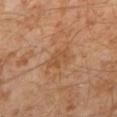workup: total-body-photography surveillance lesion; no biopsy
image-analysis metrics: a detector confidence of about 100 out of 100 that the crop contains a lesion
location: the left lower leg
patient: male, aged 28–32
image source: ~15 mm tile from a whole-body skin photo